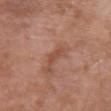{"biopsy_status": "not biopsied; imaged during a skin examination", "patient": {"sex": "female", "age_approx": 70}, "image": {"source": "total-body photography crop", "field_of_view_mm": 15}, "automated_metrics": {"vs_skin_darker_L": 8.0, "vs_skin_contrast_norm": 6.0}, "lighting": "white-light", "lesion_size": {"long_diameter_mm_approx": 2.5}, "site": "chest"}